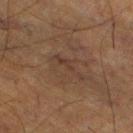Assessment:
Imaged during a routine full-body skin examination; the lesion was not biopsied and no histopathology is available.
Image and clinical context:
The total-body-photography lesion software estimated a footprint of about 6.5 mm², an eccentricity of roughly 0.9, and a symmetry-axis asymmetry near 0.5. It also reported a border-irregularity rating of about 7.5/10, a within-lesion color-variation index near 2.5/10, and radial color variation of about 0.5. It also reported a lesion-detection confidence of about 90/100. On the leg. Measured at roughly 4.5 mm in maximum diameter. A male subject roughly 60 years of age. A 15 mm close-up tile from a total-body photography series done for melanoma screening. Captured under cross-polarized illumination.